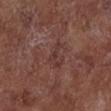workup — no biopsy performed (imaged during a skin exam)
anatomic site — the chest
subject — female, aged approximately 80
lighting — white-light illumination
size — about 3.5 mm
imaging modality — total-body-photography crop, ~15 mm field of view